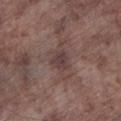| field | value |
|---|---|
| notes | imaged on a skin check; not biopsied |
| diameter | ~2.5 mm (longest diameter) |
| imaging modality | ~15 mm crop, total-body skin-cancer survey |
| site | the leg |
| illumination | white-light illumination |
| patient | male, about 75 years old |